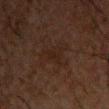Clinical impression:
The lesion was photographed on a routine skin check and not biopsied; there is no pathology result.
Image and clinical context:
The lesion is located on the front of the torso. The lesion-visualizer software estimated a mean CIELAB color near L≈16 a*≈12 b*≈17, roughly 3 lightness units darker than nearby skin, and a normalized border contrast of about 5. And it measured a classifier nevus-likeness of about 0/100 and a lesion-detection confidence of about 100/100. A roughly 15 mm field-of-view crop from a total-body skin photograph. The subject is a male in their 50s. Imaged with cross-polarized lighting. Approximately 5 mm at its widest.